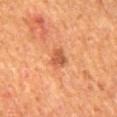workup = total-body-photography surveillance lesion; no biopsy
location = the mid back
tile lighting = cross-polarized illumination
automated lesion analysis = a footprint of about 4 mm², a shape eccentricity near 0.6, and two-axis asymmetry of about 0.3; a normalized lesion–skin contrast near 7; a border-irregularity rating of about 3/10, a within-lesion color-variation index near 2.5/10, and peripheral color asymmetry of about 1; an automated nevus-likeness rating near 70 out of 100
patient = male, in their mid-60s
image = 15 mm crop, total-body photography
lesion size = about 2.5 mm